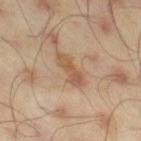subject: male, in their mid-40s
imaging modality: 15 mm crop, total-body photography
body site: the leg